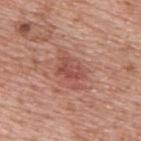| field | value |
|---|---|
| biopsy status | imaged on a skin check; not biopsied |
| image source | ~15 mm crop, total-body skin-cancer survey |
| automated metrics | a border-irregularity index near 4/10, internal color variation of about 3 on a 0–10 scale, and a peripheral color-asymmetry measure near 1 |
| lesion size | ~3 mm (longest diameter) |
| patient | male, in their mid- to late 70s |
| body site | the upper back |